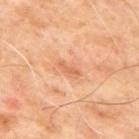The lesion was tiled from a total-body skin photograph and was not biopsied. A male patient, aged around 65. Located on the back. The tile uses cross-polarized illumination. A region of skin cropped from a whole-body photographic capture, roughly 15 mm wide. Automated tile analysis of the lesion measured an area of roughly 2.5 mm², a shape eccentricity near 0.95, and a symmetry-axis asymmetry near 0.45. And it measured a border-irregularity index near 4.5/10, a within-lesion color-variation index near 0/10, and radial color variation of about 0. Approximately 3 mm at its widest.